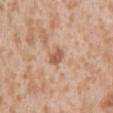Clinical impression:
Imaged during a routine full-body skin examination; the lesion was not biopsied and no histopathology is available.
Acquisition and patient details:
The lesion's longest dimension is about 2.5 mm. Automated tile analysis of the lesion measured a footprint of about 3.5 mm² and an outline eccentricity of about 0.8 (0 = round, 1 = elongated). It also reported a lesion color around L≈57 a*≈21 b*≈33 in CIELAB and a normalized lesion–skin contrast near 7.5. And it measured a color-variation rating of about 2/10. The analysis additionally found an automated nevus-likeness rating near 5 out of 100. The lesion is on the abdomen. This image is a 15 mm lesion crop taken from a total-body photograph. A male patient, aged 43 to 47.